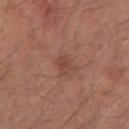This lesion was catalogued during total-body skin photography and was not selected for biopsy.